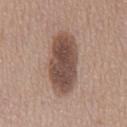The lesion was tiled from a total-body skin photograph and was not biopsied.
Cropped from a whole-body photographic skin survey; the tile spans about 15 mm.
The tile uses white-light illumination.
Longest diameter approximately 7.5 mm.
Automated tile analysis of the lesion measured an area of roughly 22 mm² and an outline eccentricity of about 0.9 (0 = round, 1 = elongated). It also reported an average lesion color of about L≈48 a*≈17 b*≈24 (CIELAB), roughly 15 lightness units darker than nearby skin, and a normalized border contrast of about 10.5.
A male patient in their mid-50s.
On the mid back.